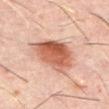biopsy_status: not biopsied; imaged during a skin examination
image:
  source: total-body photography crop
  field_of_view_mm: 15
automated_metrics:
  area_mm2_approx: 17.0
  eccentricity: 0.55
  shape_asymmetry: 0.25
  cielab_L: 54
  cielab_a: 25
  cielab_b: 30
  vs_skin_darker_L: 15.0
  vs_skin_contrast_norm: 10.0
  nevus_likeness_0_100: 100
  lesion_detection_confidence_0_100: 100
patient:
  sex: male
  age_approx: 60
site: abdomen
lighting: cross-polarized